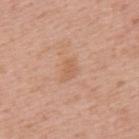The lesion was tiled from a total-body skin photograph and was not biopsied. The subject is a male aged around 60. The lesion is on the upper back. The tile uses white-light illumination. A 15 mm close-up tile from a total-body photography series done for melanoma screening.